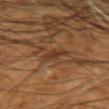Q: Was this lesion biopsied?
A: imaged on a skin check; not biopsied
Q: How was this image acquired?
A: ~15 mm crop, total-body skin-cancer survey
Q: What is the anatomic site?
A: the left arm
Q: Automated lesion metrics?
A: an area of roughly 4 mm², an outline eccentricity of about 0.75 (0 = round, 1 = elongated), and a symmetry-axis asymmetry near 0.3; a border-irregularity index near 3/10, a color-variation rating of about 1/10, and peripheral color asymmetry of about 0.5
Q: What is the lesion's diameter?
A: ~2.5 mm (longest diameter)
Q: What lighting was used for the tile?
A: cross-polarized
Q: Who is the patient?
A: male, aged approximately 60